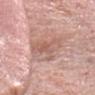biopsy status = imaged on a skin check; not biopsied | image = ~15 mm crop, total-body skin-cancer survey | patient = male, about 60 years old | size = ~6 mm (longest diameter) | image-analysis metrics = a border-irregularity rating of about 6/10, internal color variation of about 4 on a 0–10 scale, and a peripheral color-asymmetry measure near 1; an automated nevus-likeness rating near 0 out of 100 and lesion-presence confidence of about 95/100 | anatomic site = the head or neck.